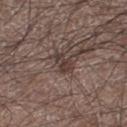{"biopsy_status": "not biopsied; imaged during a skin examination", "lesion_size": {"long_diameter_mm_approx": 3.0}, "lighting": "white-light", "patient": {"sex": "male", "age_approx": 65}, "site": "leg", "image": {"source": "total-body photography crop", "field_of_view_mm": 15}}